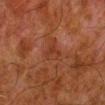Q: Was this lesion biopsied?
A: no biopsy performed (imaged during a skin exam)
Q: How was this image acquired?
A: ~15 mm crop, total-body skin-cancer survey
Q: How was the tile lit?
A: cross-polarized illumination
Q: What are the patient's age and sex?
A: male, approximately 80 years of age
Q: What is the lesion's diameter?
A: about 2.5 mm
Q: What is the anatomic site?
A: the leg
Q: Automated lesion metrics?
A: an area of roughly 4.5 mm², a shape eccentricity near 0.55, and two-axis asymmetry of about 0.35; a border-irregularity rating of about 3/10, a within-lesion color-variation index near 2.5/10, and peripheral color asymmetry of about 1